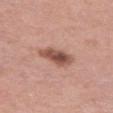{
  "biopsy_status": "not biopsied; imaged during a skin examination",
  "patient": {
    "sex": "female",
    "age_approx": 40
  },
  "lesion_size": {
    "long_diameter_mm_approx": 4.0
  },
  "image": {
    "source": "total-body photography crop",
    "field_of_view_mm": 15
  },
  "site": "left thigh",
  "lighting": "white-light"
}